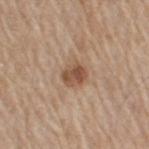workup = total-body-photography surveillance lesion; no biopsy.